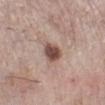follow-up = total-body-photography surveillance lesion; no biopsy | automated metrics = a lesion–skin lightness drop of about 15; a classifier nevus-likeness of about 90/100 | size = about 3 mm | acquisition = total-body-photography crop, ~15 mm field of view | site = the left lower leg | tile lighting = white-light | subject = female, aged around 65.